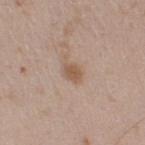* follow-up — imaged on a skin check; not biopsied
* image — total-body-photography crop, ~15 mm field of view
* site — the right upper arm
* patient — male, about 50 years old